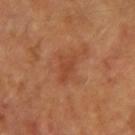follow-up = no biopsy performed (imaged during a skin exam)
subject = female, aged 58–62
image source = ~15 mm tile from a whole-body skin photo
location = the left forearm
size = ~2.5 mm (longest diameter)